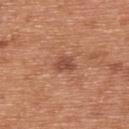Imaged during a routine full-body skin examination; the lesion was not biopsied and no histopathology is available. Automated image analysis of the tile measured a footprint of about 4 mm², an outline eccentricity of about 0.7 (0 = round, 1 = elongated), and two-axis asymmetry of about 0.3. The analysis additionally found a lesion color around L≈49 a*≈25 b*≈31 in CIELAB, roughly 10 lightness units darker than nearby skin, and a normalized border contrast of about 7. It also reported a border-irregularity rating of about 3/10, internal color variation of about 1.5 on a 0–10 scale, and peripheral color asymmetry of about 0.5. From the back. Measured at roughly 2.5 mm in maximum diameter. A close-up tile cropped from a whole-body skin photograph, about 15 mm across. A male subject in their mid- to late 60s. The tile uses white-light illumination.